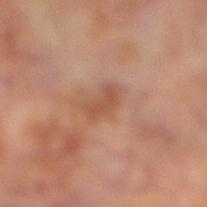Captured during whole-body skin photography for melanoma surveillance; the lesion was not biopsied. This is a cross-polarized tile. Automated tile analysis of the lesion measured an area of roughly 4 mm² and an eccentricity of roughly 0.85. The analysis additionally found a lesion color around L≈50 a*≈23 b*≈31 in CIELAB, about 8 CIELAB-L* units darker than the surrounding skin, and a lesion-to-skin contrast of about 6 (normalized; higher = more distinct). The software also gave a border-irregularity index near 3.5/10, a within-lesion color-variation index near 1.5/10, and a peripheral color-asymmetry measure near 0.5. The analysis additionally found an automated nevus-likeness rating near 0 out of 100 and lesion-presence confidence of about 100/100. The recorded lesion diameter is about 3 mm. On the right lower leg. A region of skin cropped from a whole-body photographic capture, roughly 15 mm wide. The subject is a male aged 68–72.